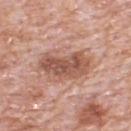notes: imaged on a skin check; not biopsied